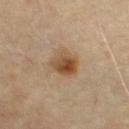notes: no biopsy performed (imaged during a skin exam)
lesion size: about 3.5 mm
image: ~15 mm tile from a whole-body skin photo
TBP lesion metrics: a mean CIELAB color near L≈47 a*≈17 b*≈32, a lesion–skin lightness drop of about 11, and a lesion-to-skin contrast of about 9.5 (normalized; higher = more distinct)
illumination: cross-polarized illumination
anatomic site: the front of the torso
patient: male, in their mid- to late 60s